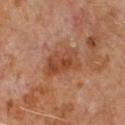{
  "biopsy_status": "not biopsied; imaged during a skin examination",
  "lighting": "cross-polarized",
  "image": {
    "source": "total-body photography crop",
    "field_of_view_mm": 15
  },
  "lesion_size": {
    "long_diameter_mm_approx": 4.5
  },
  "patient": {
    "sex": "male",
    "age_approx": 65
  },
  "site": "chest",
  "automated_metrics": {
    "vs_skin_darker_L": 9.0,
    "color_variation_0_10": 5.0,
    "peripheral_color_asymmetry": 2.0,
    "nevus_likeness_0_100": 5,
    "lesion_detection_confidence_0_100": 100
  }
}